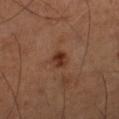Imaged during a routine full-body skin examination; the lesion was not biopsied and no histopathology is available. A male subject, aged 53–57. From the right lower leg. A 15 mm close-up tile from a total-body photography series done for melanoma screening. The recorded lesion diameter is about 2 mm.